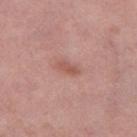{
  "biopsy_status": "not biopsied; imaged during a skin examination",
  "patient": {
    "sex": "female",
    "age_approx": 70
  },
  "automated_metrics": {
    "border_irregularity_0_10": 3.0,
    "color_variation_0_10": 0.5,
    "peripheral_color_asymmetry": 0.0,
    "nevus_likeness_0_100": 0,
    "lesion_detection_confidence_0_100": 100
  },
  "lighting": "white-light",
  "site": "leg",
  "image": {
    "source": "total-body photography crop",
    "field_of_view_mm": 15
  },
  "lesion_size": {
    "long_diameter_mm_approx": 3.0
  }
}